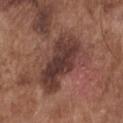| key | value |
|---|---|
| patient | male, aged 73 to 77 |
| location | the chest |
| tile lighting | white-light |
| lesion diameter | ≈8 mm |
| imaging modality | ~15 mm tile from a whole-body skin photo |